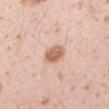This is a white-light tile. The lesion's longest dimension is about 3 mm. A 15 mm close-up tile from a total-body photography series done for melanoma screening. A female patient, in their 20s. On the left forearm.